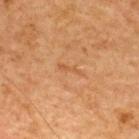Q: How large is the lesion?
A: ~3 mm (longest diameter)
Q: What did automated image analysis measure?
A: a lesion–skin lightness drop of about 5 and a lesion-to-skin contrast of about 5 (normalized; higher = more distinct)
Q: How was the tile lit?
A: cross-polarized
Q: Patient demographics?
A: male, aged 63 to 67
Q: What is the imaging modality?
A: 15 mm crop, total-body photography
Q: Where on the body is the lesion?
A: the upper back